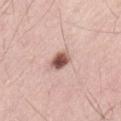Captured during whole-body skin photography for melanoma surveillance; the lesion was not biopsied. The patient is a male aged 38 to 42. A lesion tile, about 15 mm wide, cut from a 3D total-body photograph. The lesion-visualizer software estimated an average lesion color of about L≈55 a*≈22 b*≈25 (CIELAB), roughly 19 lightness units darker than nearby skin, and a normalized border contrast of about 12. It also reported border irregularity of about 1.5 on a 0–10 scale, internal color variation of about 5 on a 0–10 scale, and peripheral color asymmetry of about 1.5. It also reported an automated nevus-likeness rating near 100 out of 100 and lesion-presence confidence of about 100/100. On the left thigh. The lesion's longest dimension is about 2.5 mm. Captured under white-light illumination.